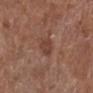The lesion was photographed on a routine skin check and not biopsied; there is no pathology result. Captured under white-light illumination. A 15 mm crop from a total-body photograph taken for skin-cancer surveillance. The lesion is on the leg. A female subject, aged 78–82. The lesion-visualizer software estimated an area of roughly 5 mm², an outline eccentricity of about 0.4 (0 = round, 1 = elongated), and two-axis asymmetry of about 0.2. The analysis additionally found a border-irregularity index near 1.5/10, internal color variation of about 2 on a 0–10 scale, and radial color variation of about 1. It also reported a detector confidence of about 100 out of 100 that the crop contains a lesion.